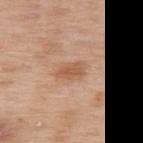Part of a total-body skin-imaging series; this lesion was reviewed on a skin check and was not flagged for biopsy. Located on the upper back. A female subject roughly 50 years of age. Cropped from a total-body skin-imaging series; the visible field is about 15 mm.